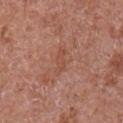workup: imaged on a skin check; not biopsied | location: the right upper arm | subject: male, about 65 years old | acquisition: ~15 mm crop, total-body skin-cancer survey | lighting: white-light | automated metrics: an automated nevus-likeness rating near 0 out of 100 | diameter: about 3 mm.